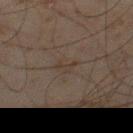Part of a total-body skin-imaging series; this lesion was reviewed on a skin check and was not flagged for biopsy. Measured at roughly 2.5 mm in maximum diameter. The subject is a male aged 43 to 47. The lesion is on the right thigh. Cropped from a whole-body photographic skin survey; the tile spans about 15 mm. Imaged with cross-polarized lighting.